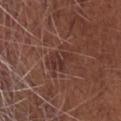subject = male, approximately 70 years of age; image = ~15 mm crop, total-body skin-cancer survey; body site = the head or neck.